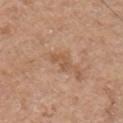Case summary:
– biopsy status: imaged on a skin check; not biopsied
– body site: the chest
– illumination: white-light illumination
– acquisition: 15 mm crop, total-body photography
– TBP lesion metrics: a lesion area of about 4 mm², a shape eccentricity near 0.75, and two-axis asymmetry of about 0.4; a classifier nevus-likeness of about 0/100 and a detector confidence of about 100 out of 100 that the crop contains a lesion
– patient: male, aged around 55
– lesion diameter: about 3 mm